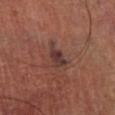The lesion was tiled from a total-body skin photograph and was not biopsied. A male subject in their 70s. Imaged with cross-polarized lighting. From the right lower leg. About 3.5 mm across. A region of skin cropped from a whole-body photographic capture, roughly 15 mm wide. The total-body-photography lesion software estimated a mean CIELAB color near L≈33 a*≈19 b*≈19 and about 9 CIELAB-L* units darker than the surrounding skin. The software also gave a border-irregularity index near 4.5/10, a within-lesion color-variation index near 3/10, and peripheral color asymmetry of about 1.